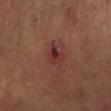biopsy status = catalogued during a skin exam; not biopsied | body site = the left lower leg | subject = male, aged 63–67 | size = about 2.5 mm | illumination = cross-polarized | imaging modality = ~15 mm tile from a whole-body skin photo.